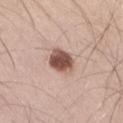Clinical impression: Recorded during total-body skin imaging; not selected for excision or biopsy. Acquisition and patient details: The patient is a male aged approximately 30. A 15 mm crop from a total-body photograph taken for skin-cancer surveillance. Captured under white-light illumination. An algorithmic analysis of the crop reported an eccentricity of roughly 0.6. The analysis additionally found a mean CIELAB color near L≈52 a*≈20 b*≈25, a lesion–skin lightness drop of about 18, and a normalized lesion–skin contrast near 12. The recorded lesion diameter is about 3.5 mm. Located on the right thigh.